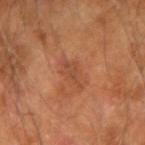| feature | finding |
|---|---|
| biopsy status | total-body-photography surveillance lesion; no biopsy |
| imaging modality | 15 mm crop, total-body photography |
| illumination | cross-polarized |
| anatomic site | the left forearm |
| patient | male, aged 63 to 67 |